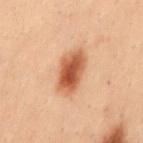Q: Is there a histopathology result?
A: imaged on a skin check; not biopsied
Q: What is the anatomic site?
A: the mid back
Q: How was the tile lit?
A: cross-polarized illumination
Q: How was this image acquired?
A: ~15 mm crop, total-body skin-cancer survey
Q: What are the patient's age and sex?
A: male, aged 28–32